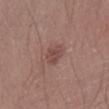Assessment:
Recorded during total-body skin imaging; not selected for excision or biopsy.
Clinical summary:
The patient is a male roughly 20 years of age. The lesion is located on the left lower leg. A close-up tile cropped from a whole-body skin photograph, about 15 mm across.